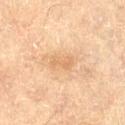Assessment: Recorded during total-body skin imaging; not selected for excision or biopsy. Acquisition and patient details: The lesion is on the abdomen. A female patient, aged 78 to 82. This image is a 15 mm lesion crop taken from a total-body photograph. The recorded lesion diameter is about 3 mm. Captured under cross-polarized illumination.